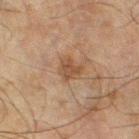Findings:
* biopsy status: imaged on a skin check; not biopsied
* patient: male, in their mid- to late 40s
* image: 15 mm crop, total-body photography
* lesion diameter: ≈2.5 mm
* site: the leg
* lighting: cross-polarized